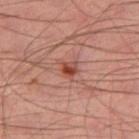automated metrics: a border-irregularity index near 2.5/10 and a color-variation rating of about 5.5/10 | acquisition: total-body-photography crop, ~15 mm field of view | subject: male, aged approximately 45 | anatomic site: the left thigh | illumination: cross-polarized illumination | lesion size: ≈2 mm.